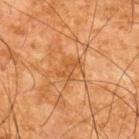Q: Was a biopsy performed?
A: total-body-photography surveillance lesion; no biopsy
Q: What are the patient's age and sex?
A: male, in their mid- to late 60s
Q: How was the tile lit?
A: cross-polarized
Q: What is the anatomic site?
A: the back
Q: What is the imaging modality?
A: 15 mm crop, total-body photography
Q: Lesion size?
A: ~3 mm (longest diameter)